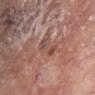Q: Is there a histopathology result?
A: total-body-photography surveillance lesion; no biopsy
Q: What kind of image is this?
A: ~15 mm tile from a whole-body skin photo
Q: What are the patient's age and sex?
A: female, aged 73–77
Q: How large is the lesion?
A: ≈3.5 mm
Q: Lesion location?
A: the chest